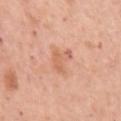Q: Is there a histopathology result?
A: catalogued during a skin exam; not biopsied
Q: Lesion location?
A: the left upper arm
Q: How was this image acquired?
A: 15 mm crop, total-body photography
Q: What are the patient's age and sex?
A: female, aged 63–67